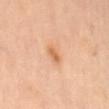notes: imaged on a skin check; not biopsied | patient: female, in their 60s | lesion diameter: about 2.5 mm | image source: 15 mm crop, total-body photography | site: the mid back | automated lesion analysis: a lesion area of about 2.5 mm² and an eccentricity of roughly 0.9; about 10 CIELAB-L* units darker than the surrounding skin and a lesion-to-skin contrast of about 7 (normalized; higher = more distinct); a border-irregularity index near 2.5/10 and a color-variation rating of about 0/10 | illumination: cross-polarized illumination.